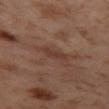notes: total-body-photography surveillance lesion; no biopsy | site: the left thigh | image source: ~15 mm tile from a whole-body skin photo | lighting: cross-polarized | patient: female, aged approximately 55 | image-analysis metrics: a mean CIELAB color near L≈38 a*≈20 b*≈26, a lesion–skin lightness drop of about 6, and a lesion-to-skin contrast of about 5.5 (normalized; higher = more distinct) | lesion diameter: ≈3 mm.